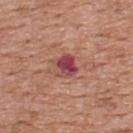| field | value |
|---|---|
| automated metrics | a nevus-likeness score of about 0/100 and a lesion-detection confidence of about 100/100 |
| imaging modality | 15 mm crop, total-body photography |
| subject | male, aged around 60 |
| site | the upper back |
| tile lighting | white-light |
| lesion diameter | about 3 mm |